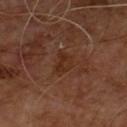Context:
From the chest. A lesion tile, about 15 mm wide, cut from a 3D total-body photograph. A male subject, aged around 55.